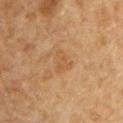Q: Was this lesion biopsied?
A: catalogued during a skin exam; not biopsied
Q: What is the anatomic site?
A: the chest
Q: What kind of image is this?
A: 15 mm crop, total-body photography
Q: What are the patient's age and sex?
A: male, roughly 60 years of age